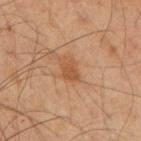Case summary:
• acquisition: 15 mm crop, total-body photography
• patient: male, aged 63 to 67
• lighting: cross-polarized illumination
• body site: the back